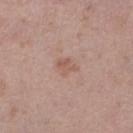  biopsy_status: not biopsied; imaged during a skin examination
  patient:
    sex: female
    age_approx: 65
  site: right lower leg
  automated_metrics:
    cielab_L: 56
    cielab_a: 20
    cielab_b: 26
    vs_skin_contrast_norm: 5.0
    nevus_likeness_0_100: 0
    lesion_detection_confidence_0_100: 100
  lesion_size:
    long_diameter_mm_approx: 2.5
  image:
    source: total-body photography crop
    field_of_view_mm: 15
  lighting: white-light